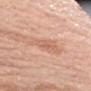A lesion tile, about 15 mm wide, cut from a 3D total-body photograph.
Located on the right upper arm.
A female subject aged 68–72.
Approximately 6 mm at its widest.
This is a white-light tile.